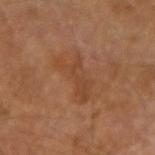This lesion was catalogued during total-body skin photography and was not selected for biopsy. This is a cross-polarized tile. A male subject aged around 65. Located on the left arm. A 15 mm close-up tile from a total-body photography series done for melanoma screening.